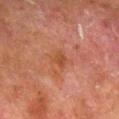No biopsy was performed on this lesion — it was imaged during a full skin examination and was not determined to be concerning. The lesion-visualizer software estimated an area of roughly 4 mm², an outline eccentricity of about 0.5 (0 = round, 1 = elongated), and two-axis asymmetry of about 0.35. It also reported border irregularity of about 3 on a 0–10 scale, internal color variation of about 1.5 on a 0–10 scale, and radial color variation of about 0.5. Measured at roughly 2.5 mm in maximum diameter. This image is a 15 mm lesion crop taken from a total-body photograph. Captured under cross-polarized illumination. The lesion is on the right lower leg. A male subject, aged 78 to 82.